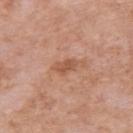biopsy_status: not biopsied; imaged during a skin examination
image:
  source: total-body photography crop
  field_of_view_mm: 15
patient:
  sex: female
  age_approx: 70
site: arm